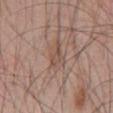* biopsy status: no biopsy performed (imaged during a skin exam)
* subject: male, approximately 45 years of age
* acquisition: 15 mm crop, total-body photography
* location: the mid back
* lesion size: about 3.5 mm
* illumination: white-light illumination